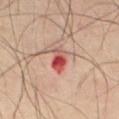A male patient about 65 years old. The tile uses cross-polarized illumination. A 15 mm crop from a total-body photograph taken for skin-cancer surveillance. The lesion-visualizer software estimated two-axis asymmetry of about 0.25. The lesion is located on the abdomen. About 3.5 mm across.